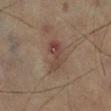notes: catalogued during a skin exam; not biopsied
acquisition: total-body-photography crop, ~15 mm field of view
location: the left lower leg
patient: male, in their 60s
tile lighting: cross-polarized illumination